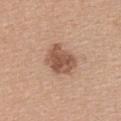Clinical impression:
Captured during whole-body skin photography for melanoma surveillance; the lesion was not biopsied.
Acquisition and patient details:
The lesion is on the back. The lesion's longest dimension is about 4 mm. A roughly 15 mm field-of-view crop from a total-body skin photograph. The total-body-photography lesion software estimated a lesion–skin lightness drop of about 13. The analysis additionally found a border-irregularity rating of about 2.5/10 and a peripheral color-asymmetry measure near 1.5. The analysis additionally found a classifier nevus-likeness of about 40/100 and a detector confidence of about 100 out of 100 that the crop contains a lesion. The patient is a female in their mid-30s. The tile uses white-light illumination.